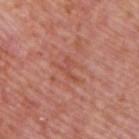The subject is a male roughly 75 years of age.
The tile uses white-light illumination.
A 15 mm close-up extracted from a 3D total-body photography capture.
The lesion is located on the back.
Approximately 3 mm at its widest.
The total-body-photography lesion software estimated a shape-asymmetry score of about 0.65 (0 = symmetric).